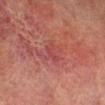Part of a total-body skin-imaging series; this lesion was reviewed on a skin check and was not flagged for biopsy. The lesion's longest dimension is about 2.5 mm. The subject is a male in their mid- to late 70s. Automated image analysis of the tile measured a lesion area of about 3 mm² and two-axis asymmetry of about 0.45. The software also gave a mean CIELAB color near L≈37 a*≈28 b*≈20, about 5 CIELAB-L* units darker than the surrounding skin, and a normalized lesion–skin contrast near 4.5. It also reported a border-irregularity index near 4.5/10, a color-variation rating of about 0/10, and peripheral color asymmetry of about 0. It also reported a nevus-likeness score of about 0/100. Imaged with cross-polarized lighting. A region of skin cropped from a whole-body photographic capture, roughly 15 mm wide. On the right lower leg.